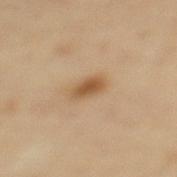The lesion was tiled from a total-body skin photograph and was not biopsied. Approximately 3 mm at its widest. This image is a 15 mm lesion crop taken from a total-body photograph. The lesion is located on the upper back. A female subject about 65 years old. Imaged with cross-polarized lighting.